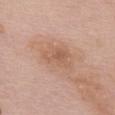Q: Was this lesion biopsied?
A: catalogued during a skin exam; not biopsied
Q: Illumination type?
A: white-light
Q: How was this image acquired?
A: ~15 mm tile from a whole-body skin photo
Q: Patient demographics?
A: female, aged 58 to 62
Q: How large is the lesion?
A: ~4.5 mm (longest diameter)
Q: What is the anatomic site?
A: the back